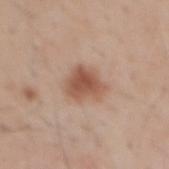Findings:
* notes: imaged on a skin check; not biopsied
* TBP lesion metrics: an area of roughly 9.5 mm², an eccentricity of roughly 0.5, and two-axis asymmetry of about 0.35; a mean CIELAB color near L≈54 a*≈21 b*≈29 and a normalized lesion–skin contrast near 8.5; a border-irregularity rating of about 3.5/10 and radial color variation of about 1; a nevus-likeness score of about 100/100
* diameter: about 4 mm
* patient: male, aged approximately 55
* image: total-body-photography crop, ~15 mm field of view
* site: the back
* tile lighting: white-light